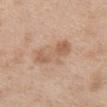• workup — imaged on a skin check; not biopsied
• patient — female, aged around 40
• lesion diameter — ≈5 mm
• site — the front of the torso
• acquisition — ~15 mm tile from a whole-body skin photo